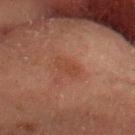notes=total-body-photography surveillance lesion; no biopsy
tile lighting=cross-polarized
patient=male, aged 58 to 62
diameter=about 3 mm
location=the head or neck
imaging modality=~15 mm crop, total-body skin-cancer survey